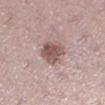Assessment:
This lesion was catalogued during total-body skin photography and was not selected for biopsy.
Context:
A female subject, in their 40s. From the leg. Captured under white-light illumination. This image is a 15 mm lesion crop taken from a total-body photograph. Measured at roughly 3.5 mm in maximum diameter.